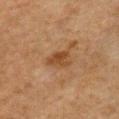Imaged during a routine full-body skin examination; the lesion was not biopsied and no histopathology is available. A male patient, in their 50s. A 15 mm close-up extracted from a 3D total-body photography capture. From the chest.